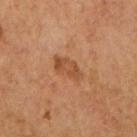Assessment:
Part of a total-body skin-imaging series; this lesion was reviewed on a skin check and was not flagged for biopsy.
Image and clinical context:
Imaged with cross-polarized lighting. A female subject, aged approximately 60. The lesion's longest dimension is about 4 mm. Located on the right upper arm. Automated image analysis of the tile measured an area of roughly 6 mm², an eccentricity of roughly 0.85, and a shape-asymmetry score of about 0.3 (0 = symmetric). The software also gave a lesion color around L≈43 a*≈21 b*≈33 in CIELAB, roughly 7 lightness units darker than nearby skin, and a normalized border contrast of about 6. The software also gave a border-irregularity rating of about 3/10, a color-variation rating of about 2.5/10, and peripheral color asymmetry of about 0.5. A 15 mm close-up tile from a total-body photography series done for melanoma screening.